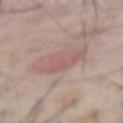Captured during whole-body skin photography for melanoma surveillance; the lesion was not biopsied. This is a white-light tile. A male subject, in their mid-60s. Approximately 5 mm at its widest. From the front of the torso. This image is a 15 mm lesion crop taken from a total-body photograph.